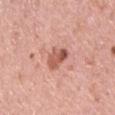Q: Was a biopsy performed?
A: total-body-photography surveillance lesion; no biopsy